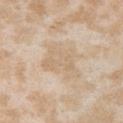This lesion was catalogued during total-body skin photography and was not selected for biopsy. A close-up tile cropped from a whole-body skin photograph, about 15 mm across. A female patient aged 23 to 27. This is a white-light tile. An algorithmic analysis of the crop reported an average lesion color of about L≈68 a*≈12 b*≈32 (CIELAB) and a lesion-to-skin contrast of about 4.5 (normalized; higher = more distinct). It also reported border irregularity of about 5 on a 0–10 scale, a within-lesion color-variation index near 2/10, and radial color variation of about 0.5. The analysis additionally found an automated nevus-likeness rating near 0 out of 100 and a lesion-detection confidence of about 100/100. The lesion is on the right upper arm.